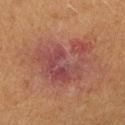{
  "biopsy_status": "not biopsied; imaged during a skin examination",
  "lighting": "cross-polarized",
  "image": {
    "source": "total-body photography crop",
    "field_of_view_mm": 15
  },
  "automated_metrics": {
    "area_mm2_approx": 25.0,
    "eccentricity": 0.45,
    "shape_asymmetry": 0.45,
    "cielab_L": 37,
    "cielab_a": 22,
    "cielab_b": 20,
    "vs_skin_contrast_norm": 7.5,
    "nevus_likeness_0_100": 0,
    "lesion_detection_confidence_0_100": 100
  },
  "site": "right forearm",
  "lesion_size": {
    "long_diameter_mm_approx": 7.0
  },
  "patient": {
    "sex": "female",
    "age_approx": 40
  }
}